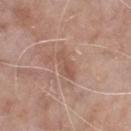{
  "biopsy_status": "not biopsied; imaged during a skin examination",
  "lighting": "white-light",
  "site": "chest",
  "patient": {
    "sex": "male",
    "age_approx": 65
  },
  "image": {
    "source": "total-body photography crop",
    "field_of_view_mm": 15
  },
  "automated_metrics": {
    "cielab_L": 54,
    "cielab_a": 20,
    "cielab_b": 27,
    "vs_skin_darker_L": 8.0,
    "vs_skin_contrast_norm": 5.5,
    "nevus_likeness_0_100": 0
  }
}